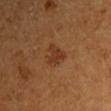<case>
<biopsy_status>not biopsied; imaged during a skin examination</biopsy_status>
<automated_metrics>
  <area_mm2_approx>5.0</area_mm2_approx>
  <shape_asymmetry>0.45</shape_asymmetry>
  <vs_skin_darker_L>7.0</vs_skin_darker_L>
  <vs_skin_contrast_norm>7.0</vs_skin_contrast_norm>
</automated_metrics>
<image>
  <source>total-body photography crop</source>
  <field_of_view_mm>15</field_of_view_mm>
</image>
<site>right upper arm</site>
<lesion_size>
  <long_diameter_mm_approx>3.0</long_diameter_mm_approx>
</lesion_size>
<patient>
  <sex>male</sex>
  <age_approx>55</age_approx>
</patient>
<lighting>cross-polarized</lighting>
</case>